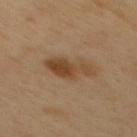biopsy_status: not biopsied; imaged during a skin examination
patient:
  sex: male
  age_approx: 50
site: back
image:
  source: total-body photography crop
  field_of_view_mm: 15
lighting: cross-polarized
lesion_size:
  long_diameter_mm_approx: 5.0